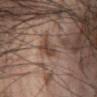site: the abdomen | patient: male, approximately 55 years of age | TBP lesion metrics: an area of roughly 4.5 mm², an eccentricity of roughly 0.75, and a shape-asymmetry score of about 0.3 (0 = symmetric); about 10 CIELAB-L* units darker than the surrounding skin and a lesion-to-skin contrast of about 8 (normalized; higher = more distinct); a color-variation rating of about 3/10 and radial color variation of about 1; a classifier nevus-likeness of about 10/100 and a detector confidence of about 95 out of 100 that the crop contains a lesion | image: ~15 mm tile from a whole-body skin photo | illumination: white-light illumination | size: ≈3 mm.